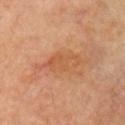workup: imaged on a skin check; not biopsied
illumination: cross-polarized illumination
image source: total-body-photography crop, ~15 mm field of view
location: the left arm
patient: male, aged approximately 65